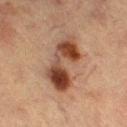The lesion was photographed on a routine skin check and not biopsied; there is no pathology result.
This is a cross-polarized tile.
Longest diameter approximately 7 mm.
A close-up tile cropped from a whole-body skin photograph, about 15 mm across.
A female subject, approximately 55 years of age.
On the leg.
The lesion-visualizer software estimated an average lesion color of about L≈35 a*≈19 b*≈25 (CIELAB) and a normalized lesion–skin contrast near 11.5.